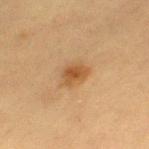No biopsy was performed on this lesion — it was imaged during a full skin examination and was not determined to be concerning.
The subject is a female aged around 55.
Imaged with cross-polarized lighting.
A 15 mm crop from a total-body photograph taken for skin-cancer surveillance.
The lesion is on the left thigh.